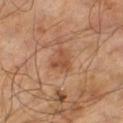Part of a total-body skin-imaging series; this lesion was reviewed on a skin check and was not flagged for biopsy. The tile uses cross-polarized illumination. Cropped from a whole-body photographic skin survey; the tile spans about 15 mm. Longest diameter approximately 3.5 mm. A male patient aged 63 to 67.